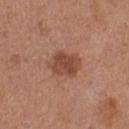This lesion was catalogued during total-body skin photography and was not selected for biopsy. Imaged with white-light lighting. A female patient aged 38–42. Cropped from a total-body skin-imaging series; the visible field is about 15 mm. From the left thigh. Approximately 4 mm at its widest. The total-body-photography lesion software estimated a shape eccentricity near 0.65 and a shape-asymmetry score of about 0.2 (0 = symmetric). And it measured an average lesion color of about L≈47 a*≈23 b*≈29 (CIELAB) and a normalized border contrast of about 7.5. The analysis additionally found an automated nevus-likeness rating near 75 out of 100 and a detector confidence of about 100 out of 100 that the crop contains a lesion.